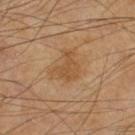The subject is a male roughly 70 years of age. An algorithmic analysis of the crop reported a mean CIELAB color near L≈49 a*≈19 b*≈36, roughly 7 lightness units darker than nearby skin, and a normalized lesion–skin contrast near 6. The analysis additionally found border irregularity of about 5.5 on a 0–10 scale and a within-lesion color-variation index near 1.5/10. And it measured an automated nevus-likeness rating near 0 out of 100 and a lesion-detection confidence of about 100/100. The lesion is on the right thigh. Measured at roughly 3.5 mm in maximum diameter. A region of skin cropped from a whole-body photographic capture, roughly 15 mm wide.